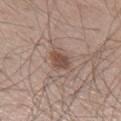biopsy_status: not biopsied; imaged during a skin examination
image:
  source: total-body photography crop
  field_of_view_mm: 15
site: right lower leg
patient:
  sex: male
  age_approx: 60